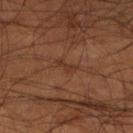The lesion was tiled from a total-body skin photograph and was not biopsied. A close-up tile cropped from a whole-body skin photograph, about 15 mm across. About 3 mm across. Located on the leg. A male subject in their mid- to late 50s.